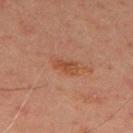Impression:
The lesion was photographed on a routine skin check and not biopsied; there is no pathology result.
Background:
The subject is a male roughly 45 years of age. The tile uses cross-polarized illumination. Longest diameter approximately 3 mm. The lesion is on the upper back. A 15 mm close-up extracted from a 3D total-body photography capture.